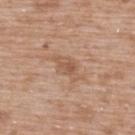biopsy_status: not biopsied; imaged during a skin examination
patient:
  sex: male
  age_approx: 50
automated_metrics:
  cielab_L: 56
  cielab_a: 19
  cielab_b: 31
  vs_skin_contrast_norm: 5.5
  nevus_likeness_0_100: 0
  lesion_detection_confidence_0_100: 100
lesion_size:
  long_diameter_mm_approx: 4.5
site: upper back
image:
  source: total-body photography crop
  field_of_view_mm: 15